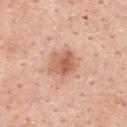Case summary:
• image — total-body-photography crop, ~15 mm field of view
• diameter — ~3.5 mm (longest diameter)
• illumination — white-light illumination
• subject — female, about 45 years old
• body site — the upper back
• automated lesion analysis — a lesion area of about 8.5 mm², a shape eccentricity near 0.45, and two-axis asymmetry of about 0.25